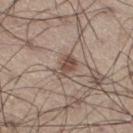Imaged during a routine full-body skin examination; the lesion was not biopsied and no histopathology is available. Automated image analysis of the tile measured a classifier nevus-likeness of about 75/100 and lesion-presence confidence of about 100/100. The lesion's longest dimension is about 3.5 mm. From the right thigh. A male patient, aged approximately 55. A 15 mm crop from a total-body photograph taken for skin-cancer surveillance.